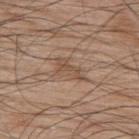No biopsy was performed on this lesion — it was imaged during a full skin examination and was not determined to be concerning.
Cropped from a whole-body photographic skin survey; the tile spans about 15 mm.
A male patient, aged 68 to 72.
The lesion is located on the upper back.